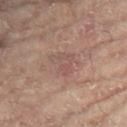This lesion was catalogued during total-body skin photography and was not selected for biopsy.
The recorded lesion diameter is about 3.5 mm.
A region of skin cropped from a whole-body photographic capture, roughly 15 mm wide.
From the left leg.
The patient is a female approximately 80 years of age.
Captured under cross-polarized illumination.
The lesion-visualizer software estimated a lesion color around L≈46 a*≈17 b*≈20 in CIELAB, about 5 CIELAB-L* units darker than the surrounding skin, and a normalized lesion–skin contrast near 4.5. And it measured a classifier nevus-likeness of about 0/100 and a lesion-detection confidence of about 100/100.